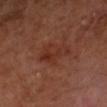The lesion was photographed on a routine skin check and not biopsied; there is no pathology result. Cropped from a whole-body photographic skin survey; the tile spans about 15 mm. A male patient approximately 55 years of age. On the arm.